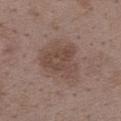biopsy status — total-body-photography surveillance lesion; no biopsy
lesion size — ~5.5 mm (longest diameter)
patient — female, aged 33 to 37
site — the upper back
imaging modality — ~15 mm crop, total-body skin-cancer survey
tile lighting — white-light